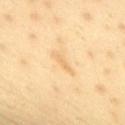diameter — about 2.5 mm
image-analysis metrics — an eccentricity of roughly 0.95 and two-axis asymmetry of about 0.35; a nevus-likeness score of about 0/100 and a lesion-detection confidence of about 95/100
lighting — cross-polarized
location — the upper back
patient — male, about 45 years old
acquisition — total-body-photography crop, ~15 mm field of view